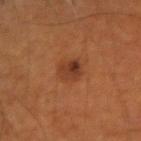The lesion was photographed on a routine skin check and not biopsied; there is no pathology result.
The lesion-visualizer software estimated a shape eccentricity near 0.55 and a shape-asymmetry score of about 0.2 (0 = symmetric). And it measured a border-irregularity index near 1.5/10, internal color variation of about 5.5 on a 0–10 scale, and peripheral color asymmetry of about 2. The analysis additionally found a nevus-likeness score of about 80/100.
A male subject, in their mid- to late 50s.
Cropped from a total-body skin-imaging series; the visible field is about 15 mm.
From the arm.